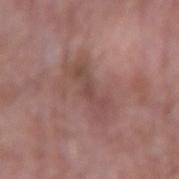Captured during whole-body skin photography for melanoma surveillance; the lesion was not biopsied.
A 15 mm close-up extracted from a 3D total-body photography capture.
A male subject, in their mid-60s.
About 5.5 mm across.
Located on the left forearm.